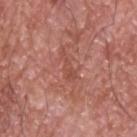notes: total-body-photography surveillance lesion; no biopsy | image source: 15 mm crop, total-body photography | automated lesion analysis: an average lesion color of about L≈49 a*≈27 b*≈28 (CIELAB), a lesion–skin lightness drop of about 7, and a lesion-to-skin contrast of about 5 (normalized; higher = more distinct); a within-lesion color-variation index near 1/10 and a peripheral color-asymmetry measure near 0.5 | subject: male, roughly 60 years of age | diameter: about 3.5 mm | anatomic site: the back.